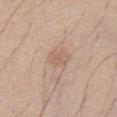<case>
  <biopsy_status>not biopsied; imaged during a skin examination</biopsy_status>
  <image>
    <source>total-body photography crop</source>
    <field_of_view_mm>15</field_of_view_mm>
  </image>
  <patient>
    <sex>male</sex>
    <age_approx>40</age_approx>
  </patient>
  <site>abdomen</site>
  <lesion_size>
    <long_diameter_mm_approx>2.5</long_diameter_mm_approx>
  </lesion_size>
</case>